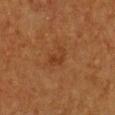Assessment:
No biopsy was performed on this lesion — it was imaged during a full skin examination and was not determined to be concerning.
Clinical summary:
The total-body-photography lesion software estimated an area of roughly 4.5 mm², a shape eccentricity near 0.8, and two-axis asymmetry of about 0.4. And it measured a mean CIELAB color near L≈31 a*≈20 b*≈30 and a lesion–skin lightness drop of about 5. It also reported a border-irregularity rating of about 4.5/10, a color-variation rating of about 1/10, and a peripheral color-asymmetry measure near 0.5. The analysis additionally found a classifier nevus-likeness of about 0/100 and lesion-presence confidence of about 100/100. Imaged with cross-polarized lighting. Measured at roughly 3 mm in maximum diameter. A female subject roughly 40 years of age. A 15 mm crop from a total-body photograph taken for skin-cancer surveillance. Located on the front of the torso.